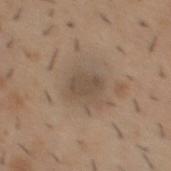workup=catalogued during a skin exam; not biopsied
image source=15 mm crop, total-body photography
location=the mid back
patient=male, approximately 40 years of age